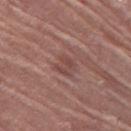Captured during whole-body skin photography for melanoma surveillance; the lesion was not biopsied.
The lesion is located on the left thigh.
Automated image analysis of the tile measured a footprint of about 5 mm², a shape eccentricity near 0.6, and two-axis asymmetry of about 0.25. And it measured a mean CIELAB color near L≈46 a*≈21 b*≈22, roughly 7 lightness units darker than nearby skin, and a normalized lesion–skin contrast near 5.5. The software also gave a nevus-likeness score of about 0/100 and lesion-presence confidence of about 70/100.
A female patient, about 80 years old.
Imaged with white-light lighting.
A 15 mm crop from a total-body photograph taken for skin-cancer surveillance.